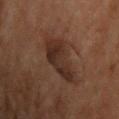Imaged during a routine full-body skin examination; the lesion was not biopsied and no histopathology is available. On the front of the torso. A male patient aged 68–72. A 15 mm close-up tile from a total-body photography series done for melanoma screening.